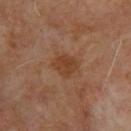• biopsy status · catalogued during a skin exam; not biopsied
• tile lighting · cross-polarized illumination
• subject · male, aged 63–67
• lesion diameter · ≈3 mm
• TBP lesion metrics · a footprint of about 7 mm² and an outline eccentricity of about 0.5 (0 = round, 1 = elongated); a lesion–skin lightness drop of about 7
• imaging modality · ~15 mm crop, total-body skin-cancer survey
• location · the upper back